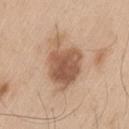Captured during whole-body skin photography for melanoma surveillance; the lesion was not biopsied. Imaged with white-light lighting. A 15 mm close-up extracted from a 3D total-body photography capture. Located on the left thigh. A male subject, aged around 60. Longest diameter approximately 6.5 mm.